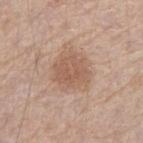Notes:
* follow-up · imaged on a skin check; not biopsied
* size · ~4.5 mm (longest diameter)
* image · total-body-photography crop, ~15 mm field of view
* anatomic site · the right forearm
* patient · male, aged 58–62